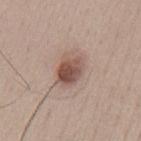Q: Is there a histopathology result?
A: total-body-photography surveillance lesion; no biopsy
Q: Lesion size?
A: ≈3.5 mm
Q: What are the patient's age and sex?
A: male, aged 38–42
Q: Lesion location?
A: the mid back
Q: How was this image acquired?
A: total-body-photography crop, ~15 mm field of view